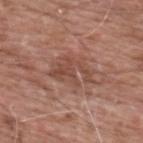<record>
<biopsy_status>not biopsied; imaged during a skin examination</biopsy_status>
<automated_metrics>
  <cielab_L>47</cielab_L>
  <cielab_a>22</cielab_a>
  <cielab_b>27</cielab_b>
  <vs_skin_contrast_norm>6.0</vs_skin_contrast_norm>
  <nevus_likeness_0_100>0</nevus_likeness_0_100>
  <lesion_detection_confidence_0_100>100</lesion_detection_confidence_0_100>
</automated_metrics>
<patient>
  <sex>male</sex>
  <age_approx>60</age_approx>
</patient>
<site>upper back</site>
<lesion_size>
  <long_diameter_mm_approx>4.5</long_diameter_mm_approx>
</lesion_size>
<image>
  <source>total-body photography crop</source>
  <field_of_view_mm>15</field_of_view_mm>
</image>
</record>